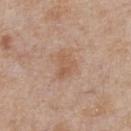| field | value |
|---|---|
| workup | no biopsy performed (imaged during a skin exam) |
| anatomic site | the chest |
| tile lighting | white-light |
| lesion size | ≈3.5 mm |
| image | 15 mm crop, total-body photography |
| patient | male, in their mid- to late 60s |